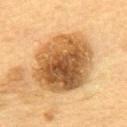Impression:
This lesion was catalogued during total-body skin photography and was not selected for biopsy.
Background:
Captured under cross-polarized illumination. The patient is a female in their 60s. On the back. The lesion's longest dimension is about 8.5 mm. A close-up tile cropped from a whole-body skin photograph, about 15 mm across.